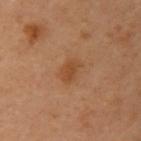<tbp_lesion>
  <biopsy_status>not biopsied; imaged during a skin examination</biopsy_status>
  <patient>
    <sex>female</sex>
    <age_approx>60</age_approx>
  </patient>
  <image>
    <source>total-body photography crop</source>
    <field_of_view_mm>15</field_of_view_mm>
  </image>
  <lesion_size>
    <long_diameter_mm_approx>3.0</long_diameter_mm_approx>
  </lesion_size>
  <site>chest</site>
</tbp_lesion>